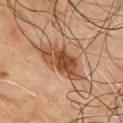Findings:
– notes · catalogued during a skin exam; not biopsied
– lesion size · ≈7 mm
– patient · male, aged around 65
– anatomic site · the front of the torso
– image · 15 mm crop, total-body photography
– illumination · cross-polarized